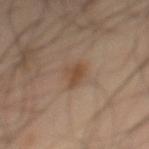Part of a total-body skin-imaging series; this lesion was reviewed on a skin check and was not flagged for biopsy. Captured under cross-polarized illumination. The recorded lesion diameter is about 3 mm. A male subject in their 50s. The lesion is located on the mid back. A roughly 15 mm field-of-view crop from a total-body skin photograph.